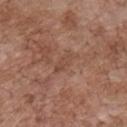Assessment: This lesion was catalogued during total-body skin photography and was not selected for biopsy. Clinical summary: On the chest. The patient is a male roughly 70 years of age. A lesion tile, about 15 mm wide, cut from a 3D total-body photograph. The lesion's longest dimension is about 3 mm. An algorithmic analysis of the crop reported an area of roughly 3 mm², a shape eccentricity near 0.9, and a shape-asymmetry score of about 0.3 (0 = symmetric). The analysis additionally found a mean CIELAB color near L≈47 a*≈21 b*≈28, a lesion–skin lightness drop of about 6, and a lesion-to-skin contrast of about 5 (normalized; higher = more distinct). And it measured border irregularity of about 3.5 on a 0–10 scale, internal color variation of about 0 on a 0–10 scale, and radial color variation of about 0. The software also gave an automated nevus-likeness rating near 0 out of 100. Imaged with white-light lighting.